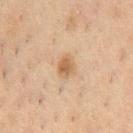Captured during whole-body skin photography for melanoma surveillance; the lesion was not biopsied. This image is a 15 mm lesion crop taken from a total-body photograph. From the chest. A male subject, aged 48–52.